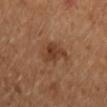Clinical impression:
Captured during whole-body skin photography for melanoma surveillance; the lesion was not biopsied.
Image and clinical context:
About 3.5 mm across. A female patient, aged 38–42. A region of skin cropped from a whole-body photographic capture, roughly 15 mm wide. The lesion is on the left arm.